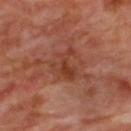image:
  source: total-body photography crop
  field_of_view_mm: 15
lighting: cross-polarized
automated_metrics:
  eccentricity: 0.6
  cielab_L: 38
  cielab_a: 26
  cielab_b: 30
  vs_skin_darker_L: 7.0
site: upper back
lesion_size:
  long_diameter_mm_approx: 3.5
patient:
  sex: male
  age_approx: 65